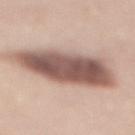No biopsy was performed on this lesion — it was imaged during a full skin examination and was not determined to be concerning.
A female subject aged 48 to 52.
Longest diameter approximately 10.5 mm.
The lesion-visualizer software estimated a lesion color around L≈55 a*≈18 b*≈23 in CIELAB and a lesion–skin lightness drop of about 18. The analysis additionally found border irregularity of about 3 on a 0–10 scale, a color-variation rating of about 6/10, and peripheral color asymmetry of about 2.
This image is a 15 mm lesion crop taken from a total-body photograph.
The tile uses white-light illumination.
Located on the back.